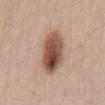This lesion was catalogued during total-body skin photography and was not selected for biopsy. About 6 mm across. Located on the chest. A female subject approximately 35 years of age. Imaged with white-light lighting. A 15 mm crop from a total-body photograph taken for skin-cancer surveillance.